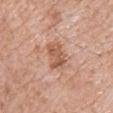Part of a total-body skin-imaging series; this lesion was reviewed on a skin check and was not flagged for biopsy. Captured under white-light illumination. The total-body-photography lesion software estimated a lesion color around L≈57 a*≈23 b*≈32 in CIELAB, a lesion–skin lightness drop of about 10, and a normalized border contrast of about 7. It also reported a border-irregularity rating of about 3/10, internal color variation of about 3 on a 0–10 scale, and radial color variation of about 1. The software also gave a nevus-likeness score of about 5/100 and a lesion-detection confidence of about 100/100. A 15 mm close-up tile from a total-body photography series done for melanoma screening. The subject is a male roughly 70 years of age. The lesion is located on the chest. About 3 mm across.